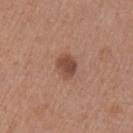workup — imaged on a skin check; not biopsied | subject — female, aged around 35 | anatomic site — the left upper arm | imaging modality — ~15 mm crop, total-body skin-cancer survey | illumination — white-light illumination.